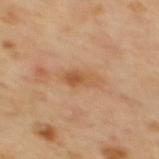Imaged during a routine full-body skin examination; the lesion was not biopsied and no histopathology is available.
Automated image analysis of the tile measured a lesion area of about 5 mm², an outline eccentricity of about 0.85 (0 = round, 1 = elongated), and two-axis asymmetry of about 0.2.
A female patient, aged approximately 55.
About 3.5 mm across.
Captured under cross-polarized illumination.
A close-up tile cropped from a whole-body skin photograph, about 15 mm across.
The lesion is located on the back.